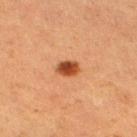The lesion was photographed on a routine skin check and not biopsied; there is no pathology result.